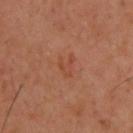No biopsy was performed on this lesion — it was imaged during a full skin examination and was not determined to be concerning.
Captured under cross-polarized illumination.
An algorithmic analysis of the crop reported a footprint of about 3.5 mm² and a shape eccentricity near 0.65. And it measured a border-irregularity index near 6.5/10, internal color variation of about 0.5 on a 0–10 scale, and radial color variation of about 0.
From the back.
Cropped from a total-body skin-imaging series; the visible field is about 15 mm.
The subject is a male approximately 40 years of age.
About 2.5 mm across.